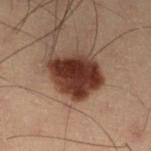Clinical impression:
This lesion was catalogued during total-body skin photography and was not selected for biopsy.
Clinical summary:
A lesion tile, about 15 mm wide, cut from a 3D total-body photograph. On the left lower leg. A male patient, about 55 years old.